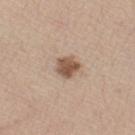Captured during whole-body skin photography for melanoma surveillance; the lesion was not biopsied.
Cropped from a whole-body photographic skin survey; the tile spans about 15 mm.
A female subject aged approximately 60.
Captured under white-light illumination.
The lesion is located on the right thigh.
An algorithmic analysis of the crop reported an area of roughly 6 mm². The software also gave a lesion color around L≈54 a*≈17 b*≈28 in CIELAB, about 14 CIELAB-L* units darker than the surrounding skin, and a normalized border contrast of about 9.5. And it measured border irregularity of about 2 on a 0–10 scale and a within-lesion color-variation index near 3.5/10. The analysis additionally found an automated nevus-likeness rating near 90 out of 100 and lesion-presence confidence of about 100/100.
About 2.5 mm across.